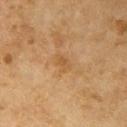Image and clinical context:
A roughly 15 mm field-of-view crop from a total-body skin photograph. This is a cross-polarized tile. The lesion is located on the right upper arm. A female patient, in their 60s.